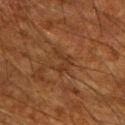This lesion was catalogued during total-body skin photography and was not selected for biopsy. The tile uses cross-polarized illumination. Measured at roughly 3 mm in maximum diameter. Cropped from a whole-body photographic skin survey; the tile spans about 15 mm. A male patient, in their mid-60s. Located on the right upper arm. Automated image analysis of the tile measured a mean CIELAB color near L≈27 a*≈18 b*≈27, about 5 CIELAB-L* units darker than the surrounding skin, and a normalized lesion–skin contrast near 5.5. The analysis additionally found a border-irregularity rating of about 7.5/10, a within-lesion color-variation index near 0/10, and radial color variation of about 0.